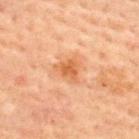This is a cross-polarized tile. The total-body-photography lesion software estimated a lesion color around L≈51 a*≈23 b*≈36 in CIELAB, a lesion–skin lightness drop of about 8, and a normalized lesion–skin contrast near 7. And it measured an automated nevus-likeness rating near 0 out of 100 and lesion-presence confidence of about 100/100. The lesion's longest dimension is about 3 mm. The lesion is located on the upper back. A 15 mm crop from a total-body photograph taken for skin-cancer surveillance. The subject is a male roughly 60 years of age.